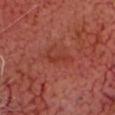Q: Is there a histopathology result?
A: total-body-photography surveillance lesion; no biopsy